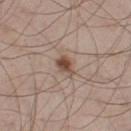Assessment: Captured during whole-body skin photography for melanoma surveillance; the lesion was not biopsied. Clinical summary: The lesion is on the leg. The tile uses white-light illumination. This image is a 15 mm lesion crop taken from a total-body photograph. About 2.5 mm across. A male subject, aged 58 to 62.